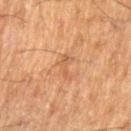image: 15 mm crop, total-body photography
size: ~3 mm (longest diameter)
patient: male, in their 60s
location: the left upper arm
automated metrics: an area of roughly 3 mm², an outline eccentricity of about 0.95 (0 = round, 1 = elongated), and two-axis asymmetry of about 0.6; a lesion color around L≈45 a*≈19 b*≈31 in CIELAB, roughly 6 lightness units darker than nearby skin, and a lesion-to-skin contrast of about 5 (normalized; higher = more distinct); peripheral color asymmetry of about 0; a nevus-likeness score of about 0/100
illumination: cross-polarized